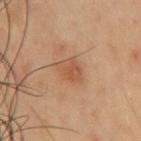Imaged during a routine full-body skin examination; the lesion was not biopsied and no histopathology is available. Cropped from a whole-body photographic skin survey; the tile spans about 15 mm. The lesion is located on the chest. The tile uses cross-polarized illumination. A male patient in their mid-50s.